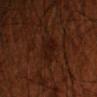Impression: Part of a total-body skin-imaging series; this lesion was reviewed on a skin check and was not flagged for biopsy. Image and clinical context: A close-up tile cropped from a whole-body skin photograph, about 15 mm across. The lesion-visualizer software estimated an area of roughly 7 mm², a shape eccentricity near 0.65, and a shape-asymmetry score of about 0.3 (0 = symmetric). The analysis additionally found a mean CIELAB color near L≈16 a*≈19 b*≈20, a lesion–skin lightness drop of about 5, and a normalized border contrast of about 7. The analysis additionally found a nevus-likeness score of about 5/100. Measured at roughly 3.5 mm in maximum diameter. The subject is a male roughly 70 years of age. Imaged with cross-polarized lighting. Located on the left forearm.